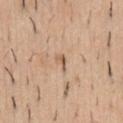Recorded during total-body skin imaging; not selected for excision or biopsy.